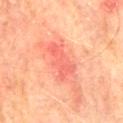Part of a total-body skin-imaging series; this lesion was reviewed on a skin check and was not flagged for biopsy.
Imaged with cross-polarized lighting.
The lesion is on the mid back.
Approximately 4.5 mm at its widest.
A male subject in their mid- to late 70s.
This image is a 15 mm lesion crop taken from a total-body photograph.